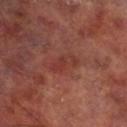body site: the leg
tile lighting: cross-polarized
imaging modality: total-body-photography crop, ~15 mm field of view
lesion size: about 4 mm
subject: male, aged around 60